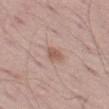Captured during whole-body skin photography for melanoma surveillance; the lesion was not biopsied. A male subject aged 53–57. The recorded lesion diameter is about 2.5 mm. Cropped from a whole-body photographic skin survey; the tile spans about 15 mm. Located on the left thigh. The tile uses white-light illumination.